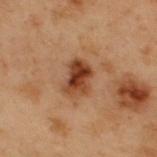  biopsy_status: not biopsied; imaged during a skin examination
  lesion_size:
    long_diameter_mm_approx: 3.5
  lighting: cross-polarized
  site: upper back
  patient:
    sex: male
    age_approx: 55
  image:
    source: total-body photography crop
    field_of_view_mm: 15